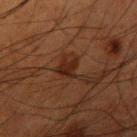Recorded during total-body skin imaging; not selected for excision or biopsy.
The lesion is located on the right upper arm.
A lesion tile, about 15 mm wide, cut from a 3D total-body photograph.
A male patient aged approximately 50.